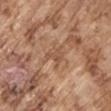This lesion was catalogued during total-body skin photography and was not selected for biopsy.
The tile uses white-light illumination.
A 15 mm close-up extracted from a 3D total-body photography capture.
Automated image analysis of the tile measured a border-irregularity index near 5.5/10 and radial color variation of about 1. It also reported an automated nevus-likeness rating near 0 out of 100 and a detector confidence of about 65 out of 100 that the crop contains a lesion.
A male patient, aged 73–77.
Located on the right upper arm.
About 2.5 mm across.